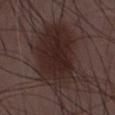Imaged during a routine full-body skin examination; the lesion was not biopsied and no histopathology is available.
The lesion's longest dimension is about 9 mm.
The total-body-photography lesion software estimated a footprint of about 44 mm², a shape eccentricity near 0.7, and a shape-asymmetry score of about 0.25 (0 = symmetric). It also reported a lesion color around L≈25 a*≈15 b*≈16 in CIELAB. And it measured an automated nevus-likeness rating near 80 out of 100 and lesion-presence confidence of about 100/100.
The subject is a male about 50 years old.
A region of skin cropped from a whole-body photographic capture, roughly 15 mm wide.